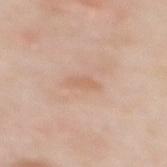Q: Is there a histopathology result?
A: total-body-photography surveillance lesion; no biopsy
Q: Lesion location?
A: the upper back
Q: What did automated image analysis measure?
A: an average lesion color of about L≈64 a*≈20 b*≈31 (CIELAB), about 6 CIELAB-L* units darker than the surrounding skin, and a normalized border contrast of about 5; a nevus-likeness score of about 0/100 and lesion-presence confidence of about 100/100
Q: Lesion size?
A: ≈3 mm
Q: What kind of image is this?
A: ~15 mm crop, total-body skin-cancer survey
Q: Who is the patient?
A: female, aged 48 to 52
Q: How was the tile lit?
A: white-light illumination